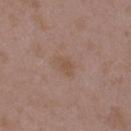Acquisition and patient details:
Approximately 2.5 mm at its widest. The patient is a female in their mid- to late 30s. Located on the arm. A roughly 15 mm field-of-view crop from a total-body skin photograph. The tile uses white-light illumination.